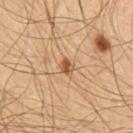Q: Was a biopsy performed?
A: catalogued during a skin exam; not biopsied
Q: What is the anatomic site?
A: the chest
Q: How was this image acquired?
A: 15 mm crop, total-body photography
Q: Patient demographics?
A: male, aged around 65